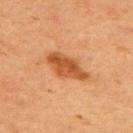Q: Was this lesion biopsied?
A: total-body-photography surveillance lesion; no biopsy
Q: Lesion location?
A: the upper back
Q: How was this image acquired?
A: ~15 mm crop, total-body skin-cancer survey
Q: Who is the patient?
A: female, roughly 50 years of age